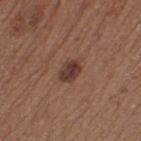Case summary:
– workup · total-body-photography surveillance lesion; no biopsy
– anatomic site · the left thigh
– lighting · white-light
– automated lesion analysis · an automated nevus-likeness rating near 75 out of 100 and lesion-presence confidence of about 100/100
– subject · female, roughly 40 years of age
– diameter · about 3 mm
– imaging modality · total-body-photography crop, ~15 mm field of view